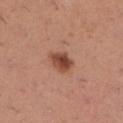Findings:
– biopsy status: total-body-photography surveillance lesion; no biopsy
– site: the leg
– patient: female, aged 28 to 32
– diameter: ≈3.5 mm
– image: 15 mm crop, total-body photography